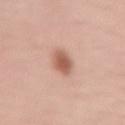No biopsy was performed on this lesion — it was imaged during a full skin examination and was not determined to be concerning. The subject is a female aged 48 to 52. A lesion tile, about 15 mm wide, cut from a 3D total-body photograph. Located on the left upper arm.